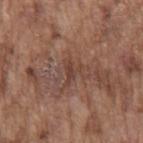Case summary:
• biopsy status: catalogued during a skin exam; not biopsied
• image: total-body-photography crop, ~15 mm field of view
• site: the mid back
• lesion size: ≈3 mm
• subject: male, roughly 75 years of age
• tile lighting: white-light illumination